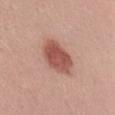Part of a total-body skin-imaging series; this lesion was reviewed on a skin check and was not flagged for biopsy.